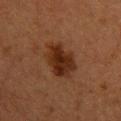Recorded during total-body skin imaging; not selected for excision or biopsy.
Automated tile analysis of the lesion measured border irregularity of about 2.5 on a 0–10 scale and internal color variation of about 3.5 on a 0–10 scale. And it measured an automated nevus-likeness rating near 85 out of 100 and a lesion-detection confidence of about 100/100.
About 4.5 mm across.
A lesion tile, about 15 mm wide, cut from a 3D total-body photograph.
A female subject, aged 38 to 42.
The lesion is located on the upper back.